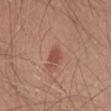No biopsy was performed on this lesion — it was imaged during a full skin examination and was not determined to be concerning.
The subject is a male aged 28 to 32.
Captured under white-light illumination.
From the left thigh.
A lesion tile, about 15 mm wide, cut from a 3D total-body photograph.